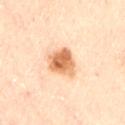Findings:
* diameter — about 3.5 mm
* subject — female, aged 58–62
* site — the lower back
* imaging modality — ~15 mm crop, total-body skin-cancer survey